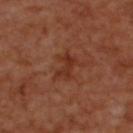Part of a total-body skin-imaging series; this lesion was reviewed on a skin check and was not flagged for biopsy.
About 3 mm across.
From the upper back.
A 15 mm crop from a total-body photograph taken for skin-cancer surveillance.
A male subject approximately 50 years of age.
Imaged with cross-polarized lighting.